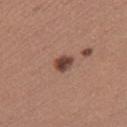Q: Was this lesion biopsied?
A: imaged on a skin check; not biopsied
Q: What did automated image analysis measure?
A: a lesion area of about 4 mm², an outline eccentricity of about 0.65 (0 = round, 1 = elongated), and two-axis asymmetry of about 0.3; a lesion color around L≈43 a*≈21 b*≈25 in CIELAB, about 14 CIELAB-L* units darker than the surrounding skin, and a normalized lesion–skin contrast near 11; an automated nevus-likeness rating near 85 out of 100 and a lesion-detection confidence of about 100/100
Q: What are the patient's age and sex?
A: female, aged around 30
Q: How was this image acquired?
A: ~15 mm crop, total-body skin-cancer survey
Q: Where on the body is the lesion?
A: the right thigh